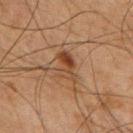image source — ~15 mm crop, total-body skin-cancer survey
location — the mid back
subject — male, roughly 65 years of age